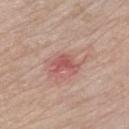A male subject, approximately 65 years of age. The lesion's longest dimension is about 3.5 mm. Located on the chest. Cropped from a total-body skin-imaging series; the visible field is about 15 mm.